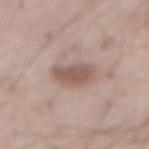Findings:
- biopsy status · catalogued during a skin exam; not biopsied
- image source · ~15 mm tile from a whole-body skin photo
- lesion size · ~4 mm (longest diameter)
- location · the front of the torso
- patient · male, in their mid-50s